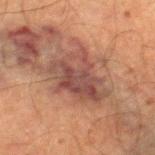No biopsy was performed on this lesion — it was imaged during a full skin examination and was not determined to be concerning. This is a cross-polarized tile. Longest diameter approximately 6 mm. A 15 mm close-up tile from a total-body photography series done for melanoma screening. A male subject, approximately 65 years of age. Located on the right thigh.